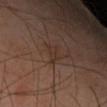Image and clinical context: A female subject, in their mid- to late 50s. From the arm. Cropped from a whole-body photographic skin survey; the tile spans about 15 mm.